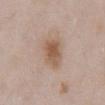Clinical impression: Imaged during a routine full-body skin examination; the lesion was not biopsied and no histopathology is available. Background: From the chest. This is a white-light tile. A roughly 15 mm field-of-view crop from a total-body skin photograph. Automated tile analysis of the lesion measured a footprint of about 9 mm², an outline eccentricity of about 0.75 (0 = round, 1 = elongated), and a shape-asymmetry score of about 0.2 (0 = symmetric). The analysis additionally found a lesion color around L≈56 a*≈17 b*≈29 in CIELAB, roughly 10 lightness units darker than nearby skin, and a lesion-to-skin contrast of about 8 (normalized; higher = more distinct). The lesion's longest dimension is about 4 mm. The subject is a male aged 53–57.